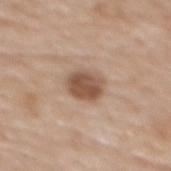<record>
<biopsy_status>not biopsied; imaged during a skin examination</biopsy_status>
<image>
  <source>total-body photography crop</source>
  <field_of_view_mm>15</field_of_view_mm>
</image>
<patient>
  <sex>male</sex>
  <age_approx>70</age_approx>
</patient>
<site>upper back</site>
</record>